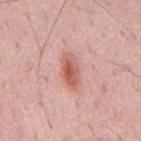follow-up = total-body-photography surveillance lesion; no biopsy
image source = ~15 mm tile from a whole-body skin photo
subject = male, about 50 years old
location = the mid back